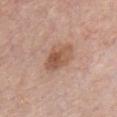Assessment:
Imaged during a routine full-body skin examination; the lesion was not biopsied and no histopathology is available.
Image and clinical context:
The tile uses white-light illumination. This image is a 15 mm lesion crop taken from a total-body photograph. From the chest. A male subject, aged around 50. Automated image analysis of the tile measured a lesion area of about 8.5 mm² and a shape eccentricity near 0.85. It also reported a lesion color around L≈54 a*≈20 b*≈30 in CIELAB and roughly 10 lightness units darker than nearby skin. The software also gave an automated nevus-likeness rating near 40 out of 100 and a lesion-detection confidence of about 100/100.